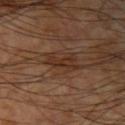  biopsy_status: not biopsied; imaged during a skin examination
  image:
    source: total-body photography crop
    field_of_view_mm: 15
  site: right upper arm
  patient:
    sex: male
    age_approx: 60
  lesion_size:
    long_diameter_mm_approx: 3.0
  lighting: cross-polarized